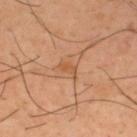Longest diameter approximately 3 mm. Automated tile analysis of the lesion measured a lesion area of about 3 mm², an outline eccentricity of about 0.85 (0 = round, 1 = elongated), and a symmetry-axis asymmetry near 0.45. The software also gave a border-irregularity index near 4.5/10, internal color variation of about 0.5 on a 0–10 scale, and a peripheral color-asymmetry measure near 0. It also reported a detector confidence of about 100 out of 100 that the crop contains a lesion. Captured under cross-polarized illumination. The lesion is located on the upper back. A male patient, in their 40s. A roughly 15 mm field-of-view crop from a total-body skin photograph.